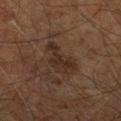Findings:
- image source — 15 mm crop, total-body photography
- patient — male, aged 58 to 62
- lesion size — about 5 mm
- tile lighting — cross-polarized illumination
- automated lesion analysis — roughly 7 lightness units darker than nearby skin and a normalized border contrast of about 7.5; a border-irregularity index near 5.5/10, a color-variation rating of about 2.5/10, and a peripheral color-asymmetry measure near 1; a classifier nevus-likeness of about 0/100
- site — the right leg